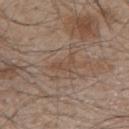Q: Is there a histopathology result?
A: no biopsy performed (imaged during a skin exam)
Q: Illumination type?
A: white-light
Q: What is the imaging modality?
A: total-body-photography crop, ~15 mm field of view
Q: What are the patient's age and sex?
A: male, aged 43 to 47
Q: How large is the lesion?
A: about 3 mm
Q: Where on the body is the lesion?
A: the mid back